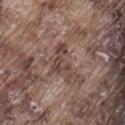Imaged during a routine full-body skin examination; the lesion was not biopsied and no histopathology is available. A male subject, approximately 75 years of age. A 15 mm close-up tile from a total-body photography series done for melanoma screening. The recorded lesion diameter is about 4 mm. The tile uses white-light illumination. On the back.